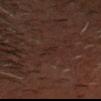notes: imaged on a skin check; not biopsied | lesion size: ~2.5 mm (longest diameter) | lighting: cross-polarized illumination | automated metrics: a lesion area of about 2 mm²; a mean CIELAB color near L≈21 a*≈15 b*≈18, a lesion–skin lightness drop of about 4, and a normalized border contrast of about 5; a border-irregularity index near 3.5/10 and a color-variation rating of about 0/10 | image: 15 mm crop, total-body photography | patient: male, approximately 60 years of age | anatomic site: the head or neck.